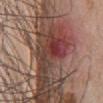{
  "site": "chest",
  "patient": {
    "sex": "male",
    "age_approx": 60
  },
  "lighting": "white-light",
  "image": {
    "source": "total-body photography crop",
    "field_of_view_mm": 15
  },
  "lesion_size": {
    "long_diameter_mm_approx": 5.0
  }
}